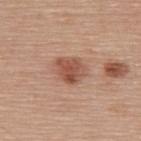{"biopsy_status": "not biopsied; imaged during a skin examination", "automated_metrics": {"nevus_likeness_0_100": 90}, "patient": {"sex": "female", "age_approx": 60}, "site": "upper back", "image": {"source": "total-body photography crop", "field_of_view_mm": 15}, "lesion_size": {"long_diameter_mm_approx": 3.0}}